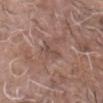{"biopsy_status": "not biopsied; imaged during a skin examination", "automated_metrics": {"cielab_L": 48, "cielab_a": 18, "cielab_b": 23, "vs_skin_contrast_norm": 5.0, "border_irregularity_0_10": 5.0, "peripheral_color_asymmetry": 0.0}, "image": {"source": "total-body photography crop", "field_of_view_mm": 15}, "lighting": "white-light", "lesion_size": {"long_diameter_mm_approx": 3.0}, "patient": {"sex": "male", "age_approx": 70}, "site": "head or neck"}